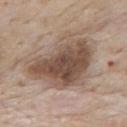Clinical impression: Recorded during total-body skin imaging; not selected for excision or biopsy. Context: Captured under white-light illumination. The lesion is on the upper back. This image is a 15 mm lesion crop taken from a total-body photograph. A male subject, aged around 85 or older. The lesion's longest dimension is about 7.5 mm.